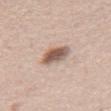Case summary:
• workup — imaged on a skin check; not biopsied
• anatomic site — the front of the torso
• acquisition — ~15 mm tile from a whole-body skin photo
• lesion size — about 3.5 mm
• patient — male, aged approximately 40
• tile lighting — white-light
• automated lesion analysis — a border-irregularity rating of about 2/10, internal color variation of about 6 on a 0–10 scale, and a peripheral color-asymmetry measure near 1.5; an automated nevus-likeness rating near 95 out of 100 and lesion-presence confidence of about 100/100